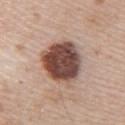The lesion is on the arm.
About 5.5 mm across.
The tile uses white-light illumination.
A region of skin cropped from a whole-body photographic capture, roughly 15 mm wide.
A female patient about 50 years old.